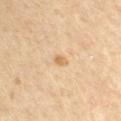This lesion was catalogued during total-body skin photography and was not selected for biopsy.
An algorithmic analysis of the crop reported a lesion area of about 2 mm², a shape eccentricity near 0.75, and a shape-asymmetry score of about 0.2 (0 = symmetric). The software also gave internal color variation of about 0.5 on a 0–10 scale and a peripheral color-asymmetry measure near 0. And it measured a classifier nevus-likeness of about 5/100 and a detector confidence of about 100 out of 100 that the crop contains a lesion.
The lesion is located on the left upper arm.
The recorded lesion diameter is about 2 mm.
Captured under cross-polarized illumination.
A male patient, roughly 55 years of age.
A roughly 15 mm field-of-view crop from a total-body skin photograph.